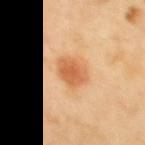workup = total-body-photography surveillance lesion; no biopsy | location = the upper back | diameter = about 4.5 mm | automated metrics = a border-irregularity index near 1.5/10 | patient = female, aged approximately 60 | image source = 15 mm crop, total-body photography | illumination = cross-polarized.